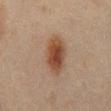Q: How was the tile lit?
A: cross-polarized
Q: Patient demographics?
A: male, aged approximately 45
Q: How large is the lesion?
A: about 5.5 mm
Q: Lesion location?
A: the abdomen
Q: What kind of image is this?
A: total-body-photography crop, ~15 mm field of view